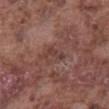Q: Was a biopsy performed?
A: total-body-photography surveillance lesion; no biopsy
Q: Automated lesion metrics?
A: an area of roughly 3 mm², a shape eccentricity near 0.9, and two-axis asymmetry of about 0.55; an average lesion color of about L≈39 a*≈20 b*≈21 (CIELAB) and a normalized border contrast of about 6; an automated nevus-likeness rating near 0 out of 100
Q: What is the anatomic site?
A: the abdomen
Q: How was this image acquired?
A: ~15 mm crop, total-body skin-cancer survey
Q: Who is the patient?
A: male, in their mid-70s
Q: How large is the lesion?
A: about 3 mm